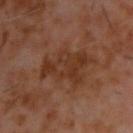biopsy_status: not biopsied; imaged during a skin examination
site: upper back
lighting: cross-polarized
image:
  source: total-body photography crop
  field_of_view_mm: 15
patient:
  sex: male
  age_approx: 60